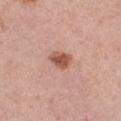{"biopsy_status": "not biopsied; imaged during a skin examination", "patient": {"sex": "female", "age_approx": 40}, "site": "left thigh", "lighting": "white-light", "image": {"source": "total-body photography crop", "field_of_view_mm": 15}, "lesion_size": {"long_diameter_mm_approx": 2.5}}